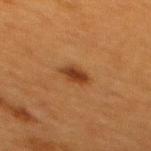workup: catalogued during a skin exam; not biopsied | tile lighting: cross-polarized illumination | patient: female, roughly 40 years of age | image source: ~15 mm tile from a whole-body skin photo | TBP lesion metrics: a footprint of about 5 mm², an outline eccentricity of about 0.75 (0 = round, 1 = elongated), and a shape-asymmetry score of about 0.15 (0 = symmetric); a lesion color around L≈33 a*≈21 b*≈31 in CIELAB, roughly 10 lightness units darker than nearby skin, and a normalized lesion–skin contrast near 9; a nevus-likeness score of about 100/100 | diameter: about 3 mm | anatomic site: the upper back.